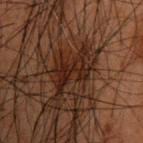Findings:
– follow-up — no biopsy performed (imaged during a skin exam)
– anatomic site — the upper back
– acquisition — 15 mm crop, total-body photography
– automated metrics — an area of roughly 8.5 mm², an outline eccentricity of about 0.9 (0 = round, 1 = elongated), and a symmetry-axis asymmetry near 0.55; border irregularity of about 9 on a 0–10 scale, a color-variation rating of about 3.5/10, and a peripheral color-asymmetry measure near 1
– patient — male, aged approximately 50